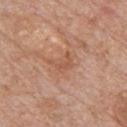biopsy status=catalogued during a skin exam; not biopsied
imaging modality=total-body-photography crop, ~15 mm field of view
lesion diameter=~3 mm (longest diameter)
subject=male, aged approximately 60
site=the chest